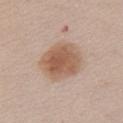workup=imaged on a skin check; not biopsied
automated metrics=a lesion area of about 18 mm², a shape eccentricity near 0.45, and a symmetry-axis asymmetry near 0.1; a nevus-likeness score of about 100/100
diameter=≈5 mm
image source=~15 mm crop, total-body skin-cancer survey
tile lighting=white-light illumination
subject=female, aged 43–47
location=the chest